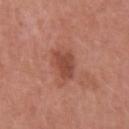Impression:
Recorded during total-body skin imaging; not selected for excision or biopsy.
Image and clinical context:
A roughly 15 mm field-of-view crop from a total-body skin photograph. The lesion-visualizer software estimated a footprint of about 7 mm² and a shape-asymmetry score of about 0.25 (0 = symmetric). And it measured a lesion–skin lightness drop of about 10 and a lesion-to-skin contrast of about 7 (normalized; higher = more distinct). The analysis additionally found border irregularity of about 2.5 on a 0–10 scale, internal color variation of about 2 on a 0–10 scale, and peripheral color asymmetry of about 0.5. It also reported an automated nevus-likeness rating near 55 out of 100 and a lesion-detection confidence of about 100/100. The subject is a female roughly 50 years of age. Imaged with white-light lighting. About 4 mm across. Located on the arm.